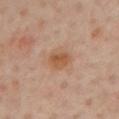Clinical impression: No biopsy was performed on this lesion — it was imaged during a full skin examination and was not determined to be concerning. Acquisition and patient details: Captured under cross-polarized illumination. Automated tile analysis of the lesion measured a footprint of about 6 mm² and a shape eccentricity near 0.55. The software also gave a detector confidence of about 100 out of 100 that the crop contains a lesion. Cropped from a total-body skin-imaging series; the visible field is about 15 mm. Longest diameter approximately 3 mm. A male subject in their mid-60s. On the chest.